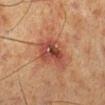Recorded during total-body skin imaging; not selected for excision or biopsy.
The lesion is on the left lower leg.
This is a cross-polarized tile.
The patient is a male approximately 60 years of age.
The lesion's longest dimension is about 4.5 mm.
The total-body-photography lesion software estimated an area of roughly 12 mm², an outline eccentricity of about 0.65 (0 = round, 1 = elongated), and a symmetry-axis asymmetry near 0.3. It also reported a border-irregularity index near 3/10, a color-variation rating of about 6/10, and peripheral color asymmetry of about 1.5.
Cropped from a total-body skin-imaging series; the visible field is about 15 mm.